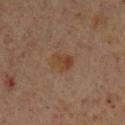A male subject aged around 60.
Imaged with cross-polarized lighting.
On the left lower leg.
About 3 mm across.
A 15 mm crop from a total-body photograph taken for skin-cancer surveillance.